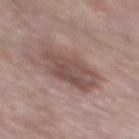{
  "biopsy_status": "not biopsied; imaged during a skin examination"
}